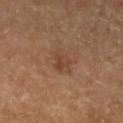The lesion was tiled from a total-body skin photograph and was not biopsied.
From the left lower leg.
A male subject about 65 years old.
A close-up tile cropped from a whole-body skin photograph, about 15 mm across.